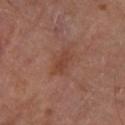biopsy status: total-body-photography surveillance lesion; no biopsy | patient: male, roughly 70 years of age | location: the left lower leg | tile lighting: cross-polarized | image: total-body-photography crop, ~15 mm field of view | lesion diameter: about 3 mm.